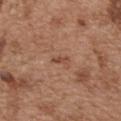{"biopsy_status": "not biopsied; imaged during a skin examination", "lighting": "white-light", "site": "front of the torso", "lesion_size": {"long_diameter_mm_approx": 2.5}, "image": {"source": "total-body photography crop", "field_of_view_mm": 15}, "patient": {"sex": "male", "age_approx": 55}}